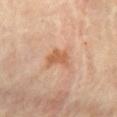biopsy_status: not biopsied; imaged during a skin examination
site: chest
image:
  source: total-body photography crop
  field_of_view_mm: 15
lighting: cross-polarized
lesion_size:
  long_diameter_mm_approx: 3.0
patient:
  sex: female
  age_approx: 60